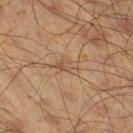<lesion>
<biopsy_status>not biopsied; imaged during a skin examination</biopsy_status>
<patient>
  <sex>male</sex>
  <age_approx>60</age_approx>
</patient>
<site>leg</site>
<image>
  <source>total-body photography crop</source>
  <field_of_view_mm>15</field_of_view_mm>
</image>
</lesion>